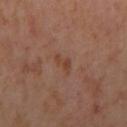{
  "biopsy_status": "not biopsied; imaged during a skin examination",
  "site": "right upper arm",
  "patient": {
    "sex": "female",
    "age_approx": 45
  },
  "image": {
    "source": "total-body photography crop",
    "field_of_view_mm": 15
  },
  "lesion_size": {
    "long_diameter_mm_approx": 2.5
  }
}